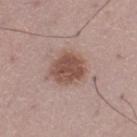follow-up: imaged on a skin check; not biopsied
lesion size: ~4.5 mm (longest diameter)
site: the leg
tile lighting: white-light
subject: male, aged around 50
image: ~15 mm tile from a whole-body skin photo